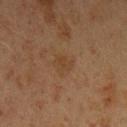Q: Was this lesion biopsied?
A: total-body-photography surveillance lesion; no biopsy
Q: How was this image acquired?
A: total-body-photography crop, ~15 mm field of view
Q: Who is the patient?
A: female, in their 20s
Q: Lesion size?
A: about 2.5 mm
Q: Where on the body is the lesion?
A: the left upper arm
Q: What did automated image analysis measure?
A: a footprint of about 4.5 mm², an eccentricity of roughly 0.6, and a shape-asymmetry score of about 0.3 (0 = symmetric); border irregularity of about 3 on a 0–10 scale and a within-lesion color-variation index near 1.5/10
Q: Illumination type?
A: cross-polarized illumination